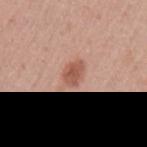The lesion was photographed on a routine skin check and not biopsied; there is no pathology result. About 3 mm across. Cropped from a whole-body photographic skin survey; the tile spans about 15 mm. The patient is a male aged approximately 45. On the arm.